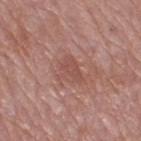notes = no biopsy performed (imaged during a skin exam); imaging modality = total-body-photography crop, ~15 mm field of view; patient = female, aged around 55; lighting = white-light illumination; anatomic site = the right thigh; size = ~3.5 mm (longest diameter).